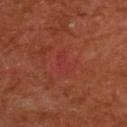Background: The subject is a male in their mid- to late 60s. About 2.5 mm across. A roughly 15 mm field-of-view crop from a total-body skin photograph. Captured under cross-polarized illumination. On the upper back. Diagnosis: Histopathology of the biopsied lesion showed a nodular basal cell carcinoma.